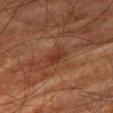workup = catalogued during a skin exam; not biopsied
anatomic site = the right thigh
imaging modality = total-body-photography crop, ~15 mm field of view
patient = male, aged approximately 80
tile lighting = cross-polarized